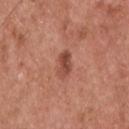  lesion_size:
    long_diameter_mm_approx: 3.0
  site: upper back
  lighting: white-light
  image:
    source: total-body photography crop
    field_of_view_mm: 15
  automated_metrics:
    eccentricity: 0.8
    shape_asymmetry: 0.25
    vs_skin_darker_L: 10.0
    vs_skin_contrast_norm: 7.5
    border_irregularity_0_10: 2.5
  patient:
    sex: male
    age_approx: 55